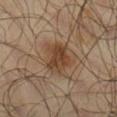- notes — imaged on a skin check; not biopsied
- acquisition — total-body-photography crop, ~15 mm field of view
- anatomic site — the right lower leg
- tile lighting — cross-polarized illumination
- TBP lesion metrics — a border-irregularity index near 3/10 and a peripheral color-asymmetry measure near 1
- subject — male, in their mid-60s